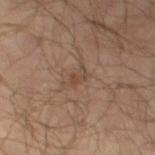No biopsy was performed on this lesion — it was imaged during a full skin examination and was not determined to be concerning.
The lesion-visualizer software estimated a border-irregularity rating of about 5.5/10 and peripheral color asymmetry of about 2. The analysis additionally found an automated nevus-likeness rating near 5 out of 100 and a detector confidence of about 100 out of 100 that the crop contains a lesion.
The lesion is located on the left thigh.
Cropped from a whole-body photographic skin survey; the tile spans about 15 mm.
The subject is a male roughly 65 years of age.
Longest diameter approximately 3 mm.